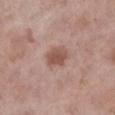The lesion was tiled from a total-body skin photograph and was not biopsied. A female subject, aged around 75. The tile uses white-light illumination. A 15 mm close-up tile from a total-body photography series done for melanoma screening. Located on the left lower leg.